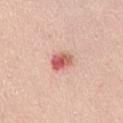Q: Is there a histopathology result?
A: total-body-photography surveillance lesion; no biopsy
Q: What is the imaging modality?
A: ~15 mm crop, total-body skin-cancer survey
Q: What are the patient's age and sex?
A: female, aged 58–62
Q: Lesion size?
A: ≈3 mm
Q: Where on the body is the lesion?
A: the abdomen